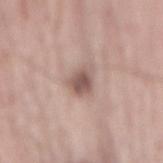Assessment: Part of a total-body skin-imaging series; this lesion was reviewed on a skin check and was not flagged for biopsy. Clinical summary: Cropped from a total-body skin-imaging series; the visible field is about 15 mm. Longest diameter approximately 3.5 mm. The lesion-visualizer software estimated a footprint of about 6.5 mm², an outline eccentricity of about 0.75 (0 = round, 1 = elongated), and a symmetry-axis asymmetry near 0.3. It also reported a border-irregularity rating of about 3/10 and a within-lesion color-variation index near 3.5/10. The subject is a male aged around 65. On the back.